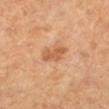workup: no biopsy performed (imaged during a skin exam).